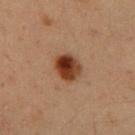Recorded during total-body skin imaging; not selected for excision or biopsy.
A 15 mm crop from a total-body photograph taken for skin-cancer surveillance.
A male patient aged around 50.
Located on the chest.
The recorded lesion diameter is about 3.5 mm.